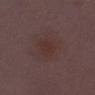The lesion is located on the right lower leg.
A 15 mm close-up extracted from a 3D total-body photography capture.
About 3 mm across.
The patient is a female aged approximately 30.
Imaged with white-light lighting.